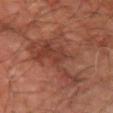The lesion was tiled from a total-body skin photograph and was not biopsied. A lesion tile, about 15 mm wide, cut from a 3D total-body photograph. A subject roughly 65 years of age. Measured at roughly 10 mm in maximum diameter. The total-body-photography lesion software estimated a lesion area of about 28 mm², an outline eccentricity of about 0.85 (0 = round, 1 = elongated), and a shape-asymmetry score of about 0.65 (0 = symmetric). The analysis additionally found a mean CIELAB color near L≈41 a*≈24 b*≈28 and a lesion-to-skin contrast of about 6.5 (normalized; higher = more distinct). It also reported an automated nevus-likeness rating near 5 out of 100 and lesion-presence confidence of about 95/100. Located on the left forearm.